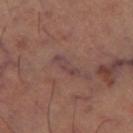{"lesion_size": {"long_diameter_mm_approx": 3.5}, "automated_metrics": {"area_mm2_approx": 4.0, "eccentricity": 0.95, "nevus_likeness_0_100": 0, "lesion_detection_confidence_0_100": 70}, "image": {"source": "total-body photography crop", "field_of_view_mm": 15}, "site": "left thigh", "lighting": "cross-polarized"}